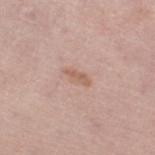notes: imaged on a skin check; not biopsied | subject: female, about 65 years old | location: the right thigh | acquisition: total-body-photography crop, ~15 mm field of view.